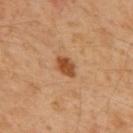<tbp_lesion>
  <biopsy_status>not biopsied; imaged during a skin examination</biopsy_status>
  <lesion_size>
    <long_diameter_mm_approx>3.0</long_diameter_mm_approx>
  </lesion_size>
  <patient>
    <sex>male</sex>
    <age_approx>60</age_approx>
  </patient>
  <lighting>cross-polarized</lighting>
  <site>upper back</site>
  <image>
    <source>total-body photography crop</source>
    <field_of_view_mm>15</field_of_view_mm>
  </image>
</tbp_lesion>